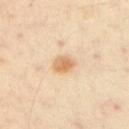Q: Was this lesion biopsied?
A: total-body-photography surveillance lesion; no biopsy
Q: What lighting was used for the tile?
A: cross-polarized illumination
Q: Lesion size?
A: ~2.5 mm (longest diameter)
Q: Who is the patient?
A: male, approximately 50 years of age
Q: How was this image acquired?
A: 15 mm crop, total-body photography
Q: What is the anatomic site?
A: the left upper arm
Q: Automated lesion metrics?
A: border irregularity of about 2 on a 0–10 scale and a within-lesion color-variation index near 4.5/10; a lesion-detection confidence of about 100/100